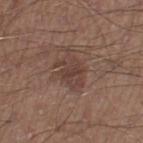The lesion was photographed on a routine skin check and not biopsied; there is no pathology result. This image is a 15 mm lesion crop taken from a total-body photograph. A male subject, aged around 50. Located on the left thigh. Captured under white-light illumination. Approximately 4 mm at its widest.